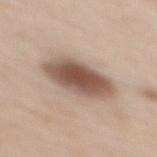Acquisition and patient details: The subject is a female about 50 years old. Located on the upper back. The lesion's longest dimension is about 6.5 mm. A region of skin cropped from a whole-body photographic capture, roughly 15 mm wide.